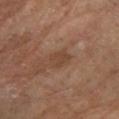Part of a total-body skin-imaging series; this lesion was reviewed on a skin check and was not flagged for biopsy. On the left lower leg. The lesion-visualizer software estimated an area of roughly 4 mm² and a symmetry-axis asymmetry near 0.25. This image is a 15 mm lesion crop taken from a total-body photograph. A female patient aged 63 to 67.